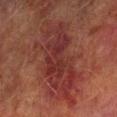Q: What is the anatomic site?
A: the left forearm
Q: How was this image acquired?
A: ~15 mm crop, total-body skin-cancer survey
Q: Who is the patient?
A: male, in their mid- to late 70s